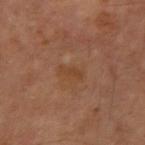Recorded during total-body skin imaging; not selected for excision or biopsy. Automated tile analysis of the lesion measured a footprint of about 2.5 mm² and a shape-asymmetry score of about 0.25 (0 = symmetric). And it measured a lesion color around L≈40 a*≈20 b*≈32 in CIELAB, a lesion–skin lightness drop of about 5, and a normalized border contrast of about 5.5. On the right upper arm. Longest diameter approximately 2.5 mm. The subject is a male aged 58–62. Imaged with cross-polarized lighting. This image is a 15 mm lesion crop taken from a total-body photograph.